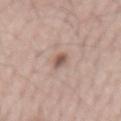  biopsy_status: not biopsied; imaged during a skin examination
  lighting: white-light
  site: mid back
  patient:
    sex: male
    age_approx: 65
  image:
    source: total-body photography crop
    field_of_view_mm: 15
  automated_metrics:
    area_mm2_approx: 3.0
    shape_asymmetry: 0.45
    border_irregularity_0_10: 4.0
    peripheral_color_asymmetry: 0.5
    nevus_likeness_0_100: 95